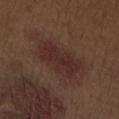  biopsy_status: not biopsied; imaged during a skin examination
  lesion_size:
    long_diameter_mm_approx: 6.0
  site: left upper arm
  image:
    source: total-body photography crop
    field_of_view_mm: 15
  patient:
    sex: male
    age_approx: 70
  automated_metrics:
    color_variation_0_10: 2.5
    peripheral_color_asymmetry: 1.0
    nevus_likeness_0_100: 65
    lesion_detection_confidence_0_100: 100